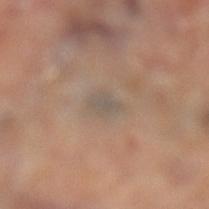Context: The lesion is on the left lower leg. A 15 mm close-up tile from a total-body photography series done for melanoma screening.